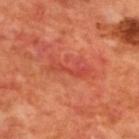Part of a total-body skin-imaging series; this lesion was reviewed on a skin check and was not flagged for biopsy. From the upper back. A region of skin cropped from a whole-body photographic capture, roughly 15 mm wide. Longest diameter approximately 4.5 mm. A male subject, about 70 years old.